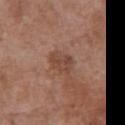Impression: No biopsy was performed on this lesion — it was imaged during a full skin examination and was not determined to be concerning. Acquisition and patient details: From the front of the torso. This image is a 15 mm lesion crop taken from a total-body photograph. The lesion-visualizer software estimated a footprint of about 5 mm², a shape eccentricity near 0.75, and a symmetry-axis asymmetry near 0.3. The software also gave a nevus-likeness score of about 0/100 and a detector confidence of about 100 out of 100 that the crop contains a lesion. The subject is a female aged approximately 75. The tile uses white-light illumination. Measured at roughly 3 mm in maximum diameter.